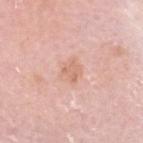<case>
  <biopsy_status>not biopsied; imaged during a skin examination</biopsy_status>
  <automated_metrics>
    <cielab_L>68</cielab_L>
    <cielab_a>22</cielab_a>
    <cielab_b>30</cielab_b>
    <vs_skin_darker_L>7.0</vs_skin_darker_L>
    <vs_skin_contrast_norm>5.5</vs_skin_contrast_norm>
  </automated_metrics>
  <image>
    <source>total-body photography crop</source>
    <field_of_view_mm>15</field_of_view_mm>
  </image>
  <lesion_size>
    <long_diameter_mm_approx>3.0</long_diameter_mm_approx>
  </lesion_size>
  <site>head or neck</site>
  <patient>
    <sex>male</sex>
    <age_approx>75</age_approx>
  </patient>
</case>